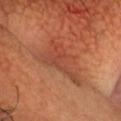Assessment:
No biopsy was performed on this lesion — it was imaged during a full skin examination and was not determined to be concerning.
Clinical summary:
Longest diameter approximately 6 mm. Cropped from a total-body skin-imaging series; the visible field is about 15 mm. The lesion-visualizer software estimated an area of roughly 14 mm², a shape eccentricity near 0.75, and two-axis asymmetry of about 0.5. The software also gave a classifier nevus-likeness of about 0/100 and a lesion-detection confidence of about 95/100. Located on the head or neck. The tile uses cross-polarized illumination. The patient is a male approximately 50 years of age.